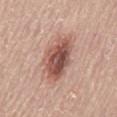Recorded during total-body skin imaging; not selected for excision or biopsy. Automated image analysis of the tile measured an average lesion color of about L≈52 a*≈23 b*≈25 (CIELAB), roughly 15 lightness units darker than nearby skin, and a normalized lesion–skin contrast near 10. It also reported a classifier nevus-likeness of about 75/100 and a detector confidence of about 100 out of 100 that the crop contains a lesion. A 15 mm crop from a total-body photograph taken for skin-cancer surveillance. The lesion is on the mid back. Captured under white-light illumination. A male patient, in their 60s.